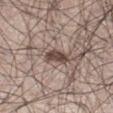workup: no biopsy performed (imaged during a skin exam); subject: male, roughly 30 years of age; body site: the leg; acquisition: 15 mm crop, total-body photography; illumination: white-light; lesion diameter: ~3.5 mm (longest diameter).